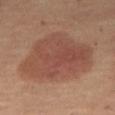imaging modality = ~15 mm tile from a whole-body skin photo; subject = female, aged 48–52; location = the right thigh; lighting = cross-polarized.